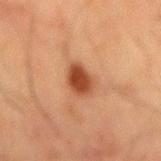notes: total-body-photography surveillance lesion; no biopsy
lesion size: ≈3.5 mm
patient: male, in their mid- to late 60s
illumination: cross-polarized
body site: the mid back
image source: ~15 mm crop, total-body skin-cancer survey